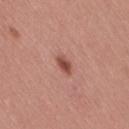{
  "biopsy_status": "not biopsied; imaged during a skin examination",
  "lesion_size": {
    "long_diameter_mm_approx": 2.5
  },
  "site": "right thigh",
  "automated_metrics": {
    "area_mm2_approx": 3.5,
    "eccentricity": 0.75,
    "vs_skin_darker_L": 12.0,
    "vs_skin_contrast_norm": 8.0,
    "border_irregularity_0_10": 2.0,
    "color_variation_0_10": 2.0,
    "peripheral_color_asymmetry": 0.5,
    "lesion_detection_confidence_0_100": 100
  },
  "lighting": "white-light",
  "image": {
    "source": "total-body photography crop",
    "field_of_view_mm": 15
  },
  "patient": {
    "sex": "female",
    "age_approx": 30
  }
}